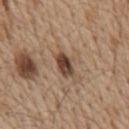biopsy status: total-body-photography surveillance lesion; no biopsy
patient: male, aged 68 to 72
size: ~3.5 mm (longest diameter)
anatomic site: the front of the torso
tile lighting: white-light illumination
image source: total-body-photography crop, ~15 mm field of view
automated metrics: a lesion color around L≈43 a*≈18 b*≈27 in CIELAB, roughly 15 lightness units darker than nearby skin, and a lesion-to-skin contrast of about 11.5 (normalized; higher = more distinct); border irregularity of about 2 on a 0–10 scale and a color-variation rating of about 6/10